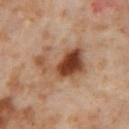Q: Was a biopsy performed?
A: no biopsy performed (imaged during a skin exam)
Q: How large is the lesion?
A: about 6 mm
Q: Who is the patient?
A: female, roughly 55 years of age
Q: What kind of image is this?
A: ~15 mm tile from a whole-body skin photo
Q: How was the tile lit?
A: cross-polarized illumination
Q: Automated lesion metrics?
A: a lesion–skin lightness drop of about 15; internal color variation of about 8.5 on a 0–10 scale and radial color variation of about 2.5; a classifier nevus-likeness of about 100/100 and lesion-presence confidence of about 100/100
Q: Lesion location?
A: the right thigh